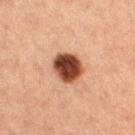The lesion was tiled from a total-body skin photograph and was not biopsied. On the leg. A female patient, in their 40s. Longest diameter approximately 3.5 mm. This is a cross-polarized tile. Cropped from a whole-body photographic skin survey; the tile spans about 15 mm.